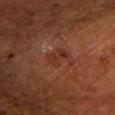The lesion was photographed on a routine skin check and not biopsied; there is no pathology result. About 2.5 mm across. The subject is a male aged approximately 80. Imaged with cross-polarized lighting. The total-body-photography lesion software estimated a lesion area of about 4.5 mm², an eccentricity of roughly 0.75, and two-axis asymmetry of about 0.35. The analysis additionally found a mean CIELAB color near L≈24 a*≈19 b*≈22 and about 5 CIELAB-L* units darker than the surrounding skin. A close-up tile cropped from a whole-body skin photograph, about 15 mm across. The lesion is located on the left lower leg.